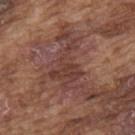Image and clinical context:
On the upper back. Automated image analysis of the tile measured an average lesion color of about L≈39 a*≈20 b*≈24 (CIELAB) and a normalized border contrast of about 6. The analysis additionally found a border-irregularity rating of about 8/10 and a peripheral color-asymmetry measure near 0.5. It also reported an automated nevus-likeness rating near 0 out of 100. The lesion's longest dimension is about 5.5 mm. A male patient in their mid-70s. Cropped from a whole-body photographic skin survey; the tile spans about 15 mm.